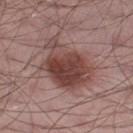No biopsy was performed on this lesion — it was imaged during a full skin examination and was not determined to be concerning.
On the left thigh.
This is a white-light tile.
An algorithmic analysis of the crop reported an average lesion color of about L≈43 a*≈21 b*≈22 (CIELAB) and a lesion–skin lightness drop of about 12.
The patient is a male aged 53–57.
A close-up tile cropped from a whole-body skin photograph, about 15 mm across.
Measured at roughly 7 mm in maximum diameter.